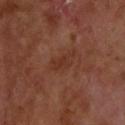{"biopsy_status": "not biopsied; imaged during a skin examination", "site": "upper back", "lesion_size": {"long_diameter_mm_approx": 3.0}, "automated_metrics": {"border_irregularity_0_10": 4.0, "color_variation_0_10": 1.0, "peripheral_color_asymmetry": 0.5, "nevus_likeness_0_100": 0, "lesion_detection_confidence_0_100": 100}, "lighting": "cross-polarized", "patient": {"sex": "male", "age_approx": 70}, "image": {"source": "total-body photography crop", "field_of_view_mm": 15}}